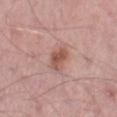subject — male, approximately 55 years of age | tile lighting — white-light illumination | lesion size — ~3.5 mm (longest diameter) | site — the right thigh | image — ~15 mm tile from a whole-body skin photo | automated lesion analysis — a lesion area of about 6 mm² and an eccentricity of roughly 0.8; a border-irregularity rating of about 2.5/10, internal color variation of about 3.5 on a 0–10 scale, and a peripheral color-asymmetry measure near 1.5; a classifier nevus-likeness of about 40/100 and a detector confidence of about 100 out of 100 that the crop contains a lesion.